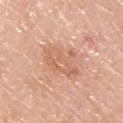No biopsy was performed on this lesion — it was imaged during a full skin examination and was not determined to be concerning. A close-up tile cropped from a whole-body skin photograph, about 15 mm across. The lesion-visualizer software estimated a mean CIELAB color near L≈64 a*≈23 b*≈33, a lesion–skin lightness drop of about 8, and a lesion-to-skin contrast of about 5.5 (normalized; higher = more distinct). It also reported an automated nevus-likeness rating near 0 out of 100 and a lesion-detection confidence of about 100/100. This is a white-light tile. The patient is a male approximately 70 years of age. The lesion is located on the back.